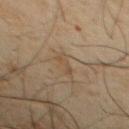Imaged during a routine full-body skin examination; the lesion was not biopsied and no histopathology is available.
A male patient roughly 60 years of age.
Approximately 2.5 mm at its widest.
A lesion tile, about 15 mm wide, cut from a 3D total-body photograph.
Automated image analysis of the tile measured an area of roughly 2.5 mm², a shape eccentricity near 0.9, and a symmetry-axis asymmetry near 0.45. It also reported an average lesion color of about L≈39 a*≈12 b*≈26 (CIELAB), a lesion–skin lightness drop of about 5, and a lesion-to-skin contrast of about 5 (normalized; higher = more distinct). The analysis additionally found border irregularity of about 5 on a 0–10 scale, internal color variation of about 0 on a 0–10 scale, and radial color variation of about 0. The software also gave a classifier nevus-likeness of about 0/100.
The lesion is on the chest.